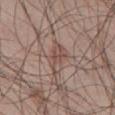{
  "biopsy_status": "not biopsied; imaged during a skin examination",
  "automated_metrics": {
    "nevus_likeness_0_100": 0,
    "lesion_detection_confidence_0_100": 50
  },
  "lighting": "white-light",
  "patient": {
    "sex": "male",
    "age_approx": 50
  },
  "lesion_size": {
    "long_diameter_mm_approx": 4.0
  },
  "site": "abdomen",
  "image": {
    "source": "total-body photography crop",
    "field_of_view_mm": 15
  }
}